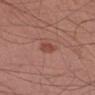Imaged during a routine full-body skin examination; the lesion was not biopsied and no histopathology is available.
About 2.5 mm across.
The patient is a male aged approximately 35.
Located on the left forearm.
A 15 mm close-up extracted from a 3D total-body photography capture.